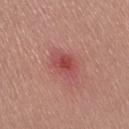<record>
<biopsy_status>not biopsied; imaged during a skin examination</biopsy_status>
<lighting>white-light</lighting>
<image>
  <source>total-body photography crop</source>
  <field_of_view_mm>15</field_of_view_mm>
</image>
<lesion_size>
  <long_diameter_mm_approx>3.5</long_diameter_mm_approx>
</lesion_size>
<site>leg</site>
<patient>
  <sex>female</sex>
  <age_approx>25</age_approx>
</patient>
<automated_metrics>
  <border_irregularity_0_10>1.5</border_irregularity_0_10>
  <color_variation_0_10>4.0</color_variation_0_10>
  <peripheral_color_asymmetry>1.0</peripheral_color_asymmetry>
</automated_metrics>
</record>